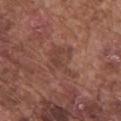This lesion was catalogued during total-body skin photography and was not selected for biopsy.
A 15 mm close-up tile from a total-body photography series done for melanoma screening.
Imaged with white-light lighting.
Automated image analysis of the tile measured a lesion area of about 10 mm², a shape eccentricity near 0.65, and a shape-asymmetry score of about 0.3 (0 = symmetric). And it measured an average lesion color of about L≈42 a*≈21 b*≈25 (CIELAB) and a normalized lesion–skin contrast near 5. And it measured border irregularity of about 5.5 on a 0–10 scale and internal color variation of about 3 on a 0–10 scale. It also reported a classifier nevus-likeness of about 0/100 and a lesion-detection confidence of about 100/100.
The subject is a male aged approximately 75.
From the chest.
The lesion's longest dimension is about 4.5 mm.